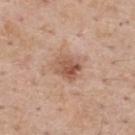biopsy status = no biopsy performed (imaged during a skin exam) | image = total-body-photography crop, ~15 mm field of view | patient = male, about 60 years old | location = the upper back.